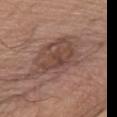No biopsy was performed on this lesion — it was imaged during a full skin examination and was not determined to be concerning. The tile uses white-light illumination. The patient is a male about 75 years old. Cropped from a whole-body photographic skin survey; the tile spans about 15 mm. The lesion's longest dimension is about 13 mm. The lesion is located on the front of the torso. An algorithmic analysis of the crop reported an average lesion color of about L≈48 a*≈18 b*≈24 (CIELAB), roughly 9 lightness units darker than nearby skin, and a lesion-to-skin contrast of about 7 (normalized; higher = more distinct).